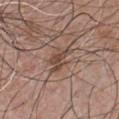| key | value |
|---|---|
| biopsy status | no biopsy performed (imaged during a skin exam) |
| location | the chest |
| image | total-body-photography crop, ~15 mm field of view |
| subject | male, aged 48–52 |